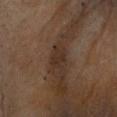No biopsy was performed on this lesion — it was imaged during a full skin examination and was not determined to be concerning. The lesion's longest dimension is about 2.5 mm. This is a cross-polarized tile. Cropped from a total-body skin-imaging series; the visible field is about 15 mm. The patient is a female in their 60s. From the head or neck.